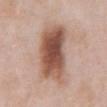Image and clinical context: A male subject about 55 years old. Captured under white-light illumination. A region of skin cropped from a whole-body photographic capture, roughly 15 mm wide. About 7.5 mm across. Located on the abdomen.